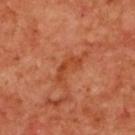Assessment:
Part of a total-body skin-imaging series; this lesion was reviewed on a skin check and was not flagged for biopsy.
Background:
A female subject approximately 40 years of age. Cropped from a total-body skin-imaging series; the visible field is about 15 mm. Located on the upper back. The tile uses cross-polarized illumination.